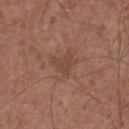| feature | finding |
|---|---|
| notes | no biopsy performed (imaged during a skin exam) |
| location | the chest |
| automated lesion analysis | an area of roughly 4 mm² and a symmetry-axis asymmetry near 0.5; a detector confidence of about 100 out of 100 that the crop contains a lesion |
| subject | male, roughly 55 years of age |
| image | ~15 mm crop, total-body skin-cancer survey |
| lesion size | about 2.5 mm |
| tile lighting | white-light illumination |